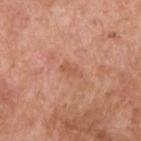Imaged during a routine full-body skin examination; the lesion was not biopsied and no histopathology is available. Cropped from a whole-body photographic skin survey; the tile spans about 15 mm. The total-body-photography lesion software estimated a classifier nevus-likeness of about 0/100 and a detector confidence of about 100 out of 100 that the crop contains a lesion. Captured under white-light illumination. The patient is a male aged 58 to 62. Longest diameter approximately 2.5 mm. On the upper back.